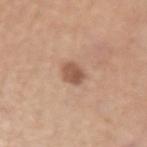follow-up — imaged on a skin check; not biopsied
lesion diameter — about 3 mm
illumination — white-light illumination
anatomic site — the arm
patient — male, aged approximately 75
image — ~15 mm crop, total-body skin-cancer survey